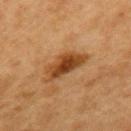biopsy status = catalogued during a skin exam; not biopsied
patient = female, in their 40s
site = the mid back
diameter = ~5 mm (longest diameter)
acquisition = ~15 mm crop, total-body skin-cancer survey